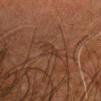<record>
<biopsy_status>not biopsied; imaged during a skin examination</biopsy_status>
<site>head or neck</site>
<patient>
  <sex>male</sex>
  <age_approx>60</age_approx>
</patient>
<image>
  <source>total-body photography crop</source>
  <field_of_view_mm>15</field_of_view_mm>
</image>
</record>